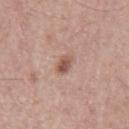This lesion was catalogued during total-body skin photography and was not selected for biopsy. Located on the back. Automated tile analysis of the lesion measured an average lesion color of about L≈54 a*≈20 b*≈27 (CIELAB), roughly 12 lightness units darker than nearby skin, and a normalized lesion–skin contrast near 8. The software also gave a border-irregularity rating of about 1.5/10 and peripheral color asymmetry of about 1.5. A male subject aged 63–67. Cropped from a whole-body photographic skin survey; the tile spans about 15 mm. Captured under white-light illumination.